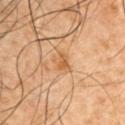From the arm. Longest diameter approximately 2.5 mm. The patient is a male aged approximately 50. Automated tile analysis of the lesion measured a footprint of about 4.5 mm², an eccentricity of roughly 0.55, and a symmetry-axis asymmetry near 0.3. It also reported a lesion color around L≈46 a*≈17 b*≈33 in CIELAB, about 6 CIELAB-L* units darker than the surrounding skin, and a normalized border contrast of about 6. It also reported internal color variation of about 3.5 on a 0–10 scale and a peripheral color-asymmetry measure near 1.5. It also reported a detector confidence of about 100 out of 100 that the crop contains a lesion. A 15 mm close-up tile from a total-body photography series done for melanoma screening. The tile uses cross-polarized illumination.